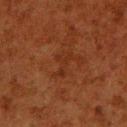biopsy status=imaged on a skin check; not biopsied
lighting=cross-polarized
patient=male, aged around 80
body site=the right lower leg
lesion size=≈3 mm
image source=~15 mm tile from a whole-body skin photo